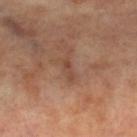Part of a total-body skin-imaging series; this lesion was reviewed on a skin check and was not flagged for biopsy. A female subject approximately 65 years of age. A 15 mm crop from a total-body photograph taken for skin-cancer surveillance. Measured at roughly 2.5 mm in maximum diameter. The lesion is on the left leg. Automated tile analysis of the lesion measured a mean CIELAB color near L≈44 a*≈20 b*≈28 and a lesion–skin lightness drop of about 7.